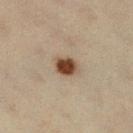Background:
Located on the right lower leg. Imaged with cross-polarized lighting. An algorithmic analysis of the crop reported an average lesion color of about L≈39 a*≈15 b*≈26 (CIELAB), about 14 CIELAB-L* units darker than the surrounding skin, and a normalized border contrast of about 12. The software also gave an automated nevus-likeness rating near 100 out of 100 and a detector confidence of about 100 out of 100 that the crop contains a lesion. This image is a 15 mm lesion crop taken from a total-body photograph. A female subject, about 65 years old.